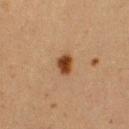Q: Was a biopsy performed?
A: total-body-photography surveillance lesion; no biopsy
Q: Patient demographics?
A: female, aged around 40
Q: Automated lesion metrics?
A: a nevus-likeness score of about 100/100 and a detector confidence of about 100 out of 100 that the crop contains a lesion
Q: What lighting was used for the tile?
A: cross-polarized
Q: What kind of image is this?
A: ~15 mm tile from a whole-body skin photo
Q: Lesion location?
A: the left upper arm
Q: What is the lesion's diameter?
A: ≈3 mm